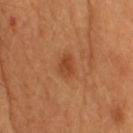Q: Was this lesion biopsied?
A: total-body-photography surveillance lesion; no biopsy